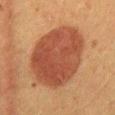{
  "biopsy_status": "not biopsied; imaged during a skin examination",
  "automated_metrics": {
    "area_mm2_approx": 46.0,
    "shape_asymmetry": 0.1,
    "cielab_L": 40,
    "cielab_a": 23,
    "cielab_b": 29,
    "vs_skin_darker_L": 11.0,
    "vs_skin_contrast_norm": 9.0,
    "nevus_likeness_0_100": 100,
    "lesion_detection_confidence_0_100": 100
  },
  "image": {
    "source": "total-body photography crop",
    "field_of_view_mm": 15
  },
  "site": "mid back",
  "patient": {
    "sex": "female",
    "age_approx": 50
  }
}